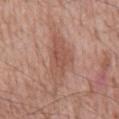The lesion was tiled from a total-body skin photograph and was not biopsied.
Automated tile analysis of the lesion measured a footprint of about 13 mm², an eccentricity of roughly 0.85, and a symmetry-axis asymmetry near 0.4. The analysis additionally found a nevus-likeness score of about 0/100 and a lesion-detection confidence of about 100/100.
A region of skin cropped from a whole-body photographic capture, roughly 15 mm wide.
The lesion is located on the mid back.
The lesion's longest dimension is about 6.5 mm.
A male patient, aged 58 to 62.
This is a white-light tile.